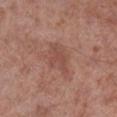The lesion was photographed on a routine skin check and not biopsied; there is no pathology result.
A male subject, in their mid- to late 50s.
The lesion is on the right lower leg.
A close-up tile cropped from a whole-body skin photograph, about 15 mm across.